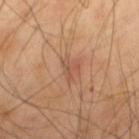{"biopsy_status": "not biopsied; imaged during a skin examination", "automated_metrics": {"border_irregularity_0_10": 6.5, "color_variation_0_10": 0.0, "peripheral_color_asymmetry": 0.0, "nevus_likeness_0_100": 0, "lesion_detection_confidence_0_100": 100}, "image": {"source": "total-body photography crop", "field_of_view_mm": 15}, "patient": {"sex": "male", "age_approx": 40}, "site": "back", "lesion_size": {"long_diameter_mm_approx": 2.5}, "lighting": "cross-polarized"}